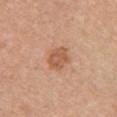Recorded during total-body skin imaging; not selected for excision or biopsy. Automated tile analysis of the lesion measured a nevus-likeness score of about 40/100 and a lesion-detection confidence of about 100/100. A roughly 15 mm field-of-view crop from a total-body skin photograph. A female subject, aged approximately 60. The tile uses white-light illumination. From the chest. Longest diameter approximately 3 mm.